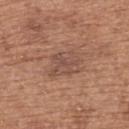The lesion was photographed on a routine skin check and not biopsied; there is no pathology result.
From the upper back.
The patient is a female in their 60s.
A close-up tile cropped from a whole-body skin photograph, about 15 mm across.